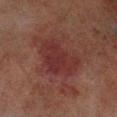Impression:
The lesion was tiled from a total-body skin photograph and was not biopsied.
Clinical summary:
Approximately 6 mm at its widest. The lesion is located on the left lower leg. A region of skin cropped from a whole-body photographic capture, roughly 15 mm wide. A male subject, approximately 70 years of age.